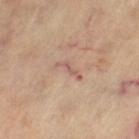workup — catalogued during a skin exam; not biopsied